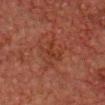No biopsy was performed on this lesion — it was imaged during a full skin examination and was not determined to be concerning.
Longest diameter approximately 2.5 mm.
The tile uses cross-polarized illumination.
On the head or neck.
The subject is a male aged approximately 60.
A lesion tile, about 15 mm wide, cut from a 3D total-body photograph.